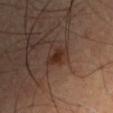| key | value |
|---|---|
| biopsy status | imaged on a skin check; not biopsied |